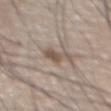Findings:
- follow-up: catalogued during a skin exam; not biopsied
- body site: the chest
- subject: male, roughly 80 years of age
- automated lesion analysis: internal color variation of about 1 on a 0–10 scale and a peripheral color-asymmetry measure near 0.5; a nevus-likeness score of about 55/100 and a detector confidence of about 90 out of 100 that the crop contains a lesion
- illumination: white-light illumination
- size: ≈3.5 mm
- acquisition: total-body-photography crop, ~15 mm field of view